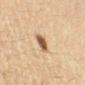<case>
  <biopsy_status>not biopsied; imaged during a skin examination</biopsy_status>
  <lesion_size>
    <long_diameter_mm_approx>3.0</long_diameter_mm_approx>
  </lesion_size>
  <patient>
    <sex>female</sex>
    <age_approx>40</age_approx>
  </patient>
  <site>right forearm</site>
  <image>
    <source>total-body photography crop</source>
    <field_of_view_mm>15</field_of_view_mm>
  </image>
  <lighting>cross-polarized</lighting>
</case>